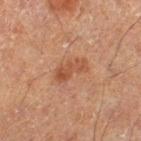Captured under cross-polarized illumination. Automated tile analysis of the lesion measured a footprint of about 6 mm² and a shape eccentricity near 0.9. And it measured a mean CIELAB color near L≈44 a*≈22 b*≈30, about 8 CIELAB-L* units darker than the surrounding skin, and a normalized border contrast of about 6.5. The analysis additionally found a border-irregularity rating of about 4/10, internal color variation of about 3 on a 0–10 scale, and peripheral color asymmetry of about 1. It also reported a classifier nevus-likeness of about 15/100 and a lesion-detection confidence of about 100/100. Approximately 4 mm at its widest. A lesion tile, about 15 mm wide, cut from a 3D total-body photograph. On the left thigh. A male patient aged approximately 65.